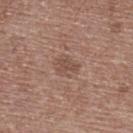Notes:
• biopsy status: total-body-photography surveillance lesion; no biopsy
• lesion diameter: ≈3 mm
• subject: male, aged 58 to 62
• image: ~15 mm tile from a whole-body skin photo
• TBP lesion metrics: an average lesion color of about L≈50 a*≈18 b*≈25 (CIELAB), about 7 CIELAB-L* units darker than the surrounding skin, and a normalized lesion–skin contrast near 5.5; a nevus-likeness score of about 0/100
• location: the upper back